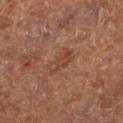Impression: The lesion was photographed on a routine skin check and not biopsied; there is no pathology result. Clinical summary: Located on the left lower leg. The patient is about 65 years old. A 15 mm close-up tile from a total-body photography series done for melanoma screening.